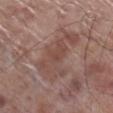biopsy status — catalogued during a skin exam; not biopsied | location — the right lower leg | patient — male, aged around 70 | lesion diameter — about 7 mm | acquisition — ~15 mm crop, total-body skin-cancer survey | automated metrics — a border-irregularity index near 6/10, a within-lesion color-variation index near 4/10, and radial color variation of about 1.